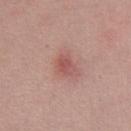On the chest. Longest diameter approximately 2.5 mm. Captured under white-light illumination. The patient is a female roughly 35 years of age. An algorithmic analysis of the crop reported a lesion area of about 4 mm², an outline eccentricity of about 0.7 (0 = round, 1 = elongated), and a symmetry-axis asymmetry near 0.25. The software also gave a mean CIELAB color near L≈53 a*≈26 b*≈23 and a normalized lesion–skin contrast near 6. The software also gave border irregularity of about 2 on a 0–10 scale, a color-variation rating of about 3/10, and radial color variation of about 1. A roughly 15 mm field-of-view crop from a total-body skin photograph.